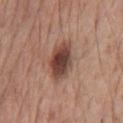Q: Was a biopsy performed?
A: total-body-photography surveillance lesion; no biopsy
Q: Who is the patient?
A: male, in their mid- to late 50s
Q: Automated lesion metrics?
A: a shape eccentricity near 0.8 and a shape-asymmetry score of about 0.15 (0 = symmetric); a within-lesion color-variation index near 7/10 and radial color variation of about 1.5; a nevus-likeness score of about 95/100 and lesion-presence confidence of about 100/100
Q: Lesion location?
A: the chest
Q: What is the imaging modality?
A: ~15 mm crop, total-body skin-cancer survey
Q: Illumination type?
A: white-light